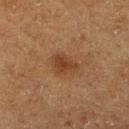Assessment:
This lesion was catalogued during total-body skin photography and was not selected for biopsy.
Background:
The lesion is located on the right lower leg. This image is a 15 mm lesion crop taken from a total-body photograph. A male patient aged around 75.